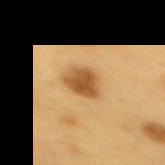No biopsy was performed on this lesion — it was imaged during a full skin examination and was not determined to be concerning.